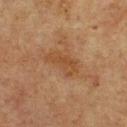follow-up = catalogued during a skin exam; not biopsied
anatomic site = the front of the torso
image = total-body-photography crop, ~15 mm field of view
patient = male, aged 73–77